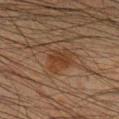<record>
<biopsy_status>not biopsied; imaged during a skin examination</biopsy_status>
<patient>
  <sex>male</sex>
  <age_approx>35</age_approx>
</patient>
<image>
  <source>total-body photography crop</source>
  <field_of_view_mm>15</field_of_view_mm>
</image>
<lesion_size>
  <long_diameter_mm_approx>3.5</long_diameter_mm_approx>
</lesion_size>
<site>right lower leg</site>
<lighting>cross-polarized</lighting>
<automated_metrics>
  <area_mm2_approx>9.0</area_mm2_approx>
  <shape_asymmetry>0.25</shape_asymmetry>
  <cielab_L>29</cielab_L>
  <cielab_a>15</cielab_a>
  <cielab_b>25</cielab_b>
  <vs_skin_darker_L>5.0</vs_skin_darker_L>
  <vs_skin_contrast_norm>6.5</vs_skin_contrast_norm>
</automated_metrics>
</record>